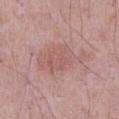Part of a total-body skin-imaging series; this lesion was reviewed on a skin check and was not flagged for biopsy. A 15 mm close-up extracted from a 3D total-body photography capture. The patient is a male aged around 70. On the front of the torso.